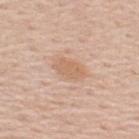Clinical impression:
This lesion was catalogued during total-body skin photography and was not selected for biopsy.
Image and clinical context:
From the back. The tile uses white-light illumination. A male subject aged 58–62. A lesion tile, about 15 mm wide, cut from a 3D total-body photograph. The total-body-photography lesion software estimated about 8 CIELAB-L* units darker than the surrounding skin and a lesion-to-skin contrast of about 6 (normalized; higher = more distinct). And it measured a border-irregularity index near 2/10 and radial color variation of about 1.